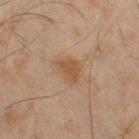Q: Is there a histopathology result?
A: catalogued during a skin exam; not biopsied
Q: Automated lesion metrics?
A: a footprint of about 6.5 mm² and a shape eccentricity near 0.75; a classifier nevus-likeness of about 70/100
Q: What is the anatomic site?
A: the right thigh
Q: What is the imaging modality?
A: total-body-photography crop, ~15 mm field of view
Q: Who is the patient?
A: male, aged around 45